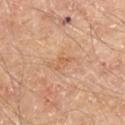| key | value |
|---|---|
| follow-up | catalogued during a skin exam; not biopsied |
| image | ~15 mm crop, total-body skin-cancer survey |
| illumination | cross-polarized illumination |
| anatomic site | the right upper arm |
| automated lesion analysis | a lesion area of about 2 mm², a shape eccentricity near 0.85, and a shape-asymmetry score of about 0.6 (0 = symmetric); a classifier nevus-likeness of about 0/100 and a detector confidence of about 100 out of 100 that the crop contains a lesion |
| subject | male, approximately 65 years of age |